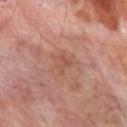Q: Was a biopsy performed?
A: imaged on a skin check; not biopsied
Q: Illumination type?
A: cross-polarized illumination
Q: Lesion size?
A: ≈2.5 mm
Q: How was this image acquired?
A: ~15 mm tile from a whole-body skin photo
Q: Automated lesion metrics?
A: an area of roughly 3 mm², an eccentricity of roughly 0.8, and a shape-asymmetry score of about 0.4 (0 = symmetric); a border-irregularity rating of about 4.5/10 and a within-lesion color-variation index near 0.5/10
Q: Who is the patient?
A: male, about 65 years old
Q: What is the anatomic site?
A: the left forearm